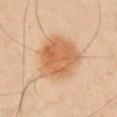This lesion was catalogued during total-body skin photography and was not selected for biopsy.
The tile uses cross-polarized illumination.
A 15 mm close-up extracted from a 3D total-body photography capture.
The patient is a male approximately 65 years of age.
From the right upper arm.
The lesion's longest dimension is about 6 mm.